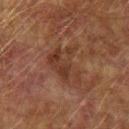| feature | finding |
|---|---|
| lesion size | about 4.5 mm |
| tile lighting | cross-polarized illumination |
| subject | male, aged approximately 75 |
| site | the arm |
| imaging modality | 15 mm crop, total-body photography |
| automated metrics | a footprint of about 11 mm² and a shape-asymmetry score of about 0.5 (0 = symmetric); a nevus-likeness score of about 0/100 and a lesion-detection confidence of about 100/100 |